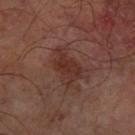{
  "biopsy_status": "not biopsied; imaged during a skin examination",
  "image": {
    "source": "total-body photography crop",
    "field_of_view_mm": 15
  },
  "site": "left forearm",
  "patient": {
    "sex": "male",
    "age_approx": 65
  },
  "lesion_size": {
    "long_diameter_mm_approx": 4.5
  },
  "automated_metrics": {
    "area_mm2_approx": 8.0,
    "eccentricity": 0.8,
    "shape_asymmetry": 0.35,
    "border_irregularity_0_10": 4.5,
    "color_variation_0_10": 3.0,
    "peripheral_color_asymmetry": 1.0
  }
}